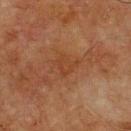This lesion was catalogued during total-body skin photography and was not selected for biopsy. This is a cross-polarized tile. The recorded lesion diameter is about 3 mm. The lesion-visualizer software estimated a lesion color around L≈33 a*≈20 b*≈29 in CIELAB, about 4 CIELAB-L* units darker than the surrounding skin, and a normalized lesion–skin contrast near 4.5. It also reported a classifier nevus-likeness of about 0/100 and lesion-presence confidence of about 100/100. From the chest. A male subject, aged approximately 75. A close-up tile cropped from a whole-body skin photograph, about 15 mm across.